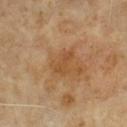No biopsy was performed on this lesion — it was imaged during a full skin examination and was not determined to be concerning.
The tile uses cross-polarized illumination.
Cropped from a whole-body photographic skin survey; the tile spans about 15 mm.
About 3.5 mm across.
From the chest.
The patient is a male aged around 60.